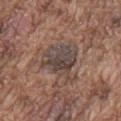{"biopsy_status": "not biopsied; imaged during a skin examination", "patient": {"sex": "male", "age_approx": 75}, "lesion_size": {"long_diameter_mm_approx": 5.5}, "image": {"source": "total-body photography crop", "field_of_view_mm": 15}, "site": "chest"}